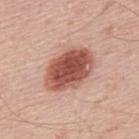| field | value |
|---|---|
| notes | catalogued during a skin exam; not biopsied |
| anatomic site | the upper back |
| image source | total-body-photography crop, ~15 mm field of view |
| patient | male, aged approximately 50 |
| illumination | white-light |